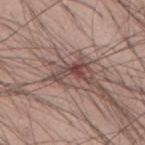Notes:
* biopsy status · total-body-photography surveillance lesion; no biopsy
* size · ~4 mm (longest diameter)
* imaging modality · 15 mm crop, total-body photography
* subject · male, approximately 60 years of age
* anatomic site · the mid back
* illumination · white-light
* automated lesion analysis · an area of roughly 5 mm², a shape eccentricity near 0.9, and a symmetry-axis asymmetry near 0.65; a lesion-to-skin contrast of about 9 (normalized; higher = more distinct); a border-irregularity rating of about 8.5/10, a within-lesion color-variation index near 2/10, and a peripheral color-asymmetry measure near 0.5; a classifier nevus-likeness of about 5/100 and a detector confidence of about 95 out of 100 that the crop contains a lesion